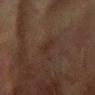Q: Is there a histopathology result?
A: no biopsy performed (imaged during a skin exam)
Q: Who is the patient?
A: male, aged approximately 75
Q: What is the imaging modality?
A: ~15 mm crop, total-body skin-cancer survey
Q: Automated lesion metrics?
A: a lesion area of about 3.5 mm², a shape eccentricity near 0.85, and a symmetry-axis asymmetry near 0.3
Q: How was the tile lit?
A: cross-polarized
Q: What is the anatomic site?
A: the right forearm